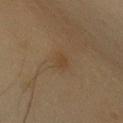Impression: Captured during whole-body skin photography for melanoma surveillance; the lesion was not biopsied. Background: The patient is a male in their mid- to late 30s. The tile uses cross-polarized illumination. This image is a 15 mm lesion crop taken from a total-body photograph. The lesion is on the front of the torso.